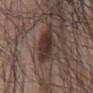follow-up — imaged on a skin check; not biopsied | body site — the mid back | subject — male, in their 70s | automated lesion analysis — a mean CIELAB color near L≈32 a*≈16 b*≈20, a lesion–skin lightness drop of about 11, and a lesion-to-skin contrast of about 10.5 (normalized; higher = more distinct) | image source — ~15 mm crop, total-body skin-cancer survey | tile lighting — white-light illumination.